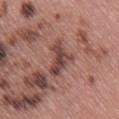| key | value |
|---|---|
| notes | total-body-photography surveillance lesion; no biopsy |
| subject | female, aged approximately 60 |
| TBP lesion metrics | a classifier nevus-likeness of about 0/100 and a lesion-detection confidence of about 95/100 |
| image | 15 mm crop, total-body photography |
| lesion size | ≈4.5 mm |
| body site | the right thigh |